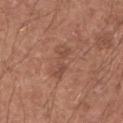Part of a total-body skin-imaging series; this lesion was reviewed on a skin check and was not flagged for biopsy.
The total-body-photography lesion software estimated an area of roughly 6 mm², an eccentricity of roughly 0.95, and two-axis asymmetry of about 0.35. It also reported a mean CIELAB color near L≈48 a*≈22 b*≈28, roughly 7 lightness units darker than nearby skin, and a normalized border contrast of about 5.
A region of skin cropped from a whole-body photographic capture, roughly 15 mm wide.
The patient is a male aged 53–57.
This is a white-light tile.
The lesion is on the arm.